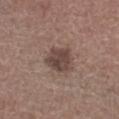A female subject in their mid-70s.
The lesion is on the right lower leg.
Captured under white-light illumination.
Approximately 3.5 mm at its widest.
A 15 mm crop from a total-body photograph taken for skin-cancer surveillance.
An algorithmic analysis of the crop reported an area of roughly 9 mm² and two-axis asymmetry of about 0.25. The analysis additionally found a lesion–skin lightness drop of about 11 and a normalized lesion–skin contrast near 8.5. The software also gave border irregularity of about 2.5 on a 0–10 scale and a within-lesion color-variation index near 3.5/10. The analysis additionally found an automated nevus-likeness rating near 25 out of 100 and a detector confidence of about 100 out of 100 that the crop contains a lesion.